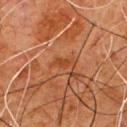– biopsy status — total-body-photography surveillance lesion; no biopsy
– body site — the front of the torso
– imaging modality — ~15 mm tile from a whole-body skin photo
– patient — male, aged 78 to 82